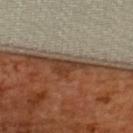The lesion was tiled from a total-body skin photograph and was not biopsied.
From the upper back.
An algorithmic analysis of the crop reported a mean CIELAB color near L≈39 a*≈21 b*≈31, about 12 CIELAB-L* units darker than the surrounding skin, and a normalized lesion–skin contrast near 10. It also reported internal color variation of about 3 on a 0–10 scale.
A female subject aged approximately 55.
This image is a 15 mm lesion crop taken from a total-body photograph.